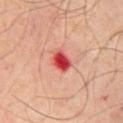Recorded during total-body skin imaging; not selected for excision or biopsy. Imaged with cross-polarized lighting. A male patient, aged approximately 70. A 15 mm close-up extracted from a 3D total-body photography capture. The recorded lesion diameter is about 3 mm. On the chest.